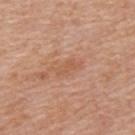{"biopsy_status": "not biopsied; imaged during a skin examination", "image": {"source": "total-body photography crop", "field_of_view_mm": 15}, "lighting": "white-light", "lesion_size": {"long_diameter_mm_approx": 3.0}, "site": "mid back", "patient": {"sex": "male", "age_approx": 60}}